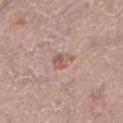Located on the left lower leg. Captured under white-light illumination. A male subject aged approximately 55. A 15 mm close-up extracted from a 3D total-body photography capture. Measured at roughly 2.5 mm in maximum diameter.